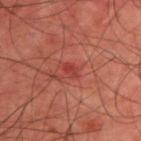Notes:
- notes · imaged on a skin check; not biopsied
- lighting · cross-polarized
- patient · male, in their mid-40s
- TBP lesion metrics · a footprint of about 2.5 mm², an outline eccentricity of about 0.8 (0 = round, 1 = elongated), and a symmetry-axis asymmetry near 0.25; a mean CIELAB color near L≈40 a*≈34 b*≈28, roughly 8 lightness units darker than nearby skin, and a lesion-to-skin contrast of about 6 (normalized; higher = more distinct); an automated nevus-likeness rating near 20 out of 100 and lesion-presence confidence of about 100/100
- location · the back
- imaging modality · total-body-photography crop, ~15 mm field of view